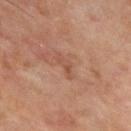This lesion was catalogued during total-body skin photography and was not selected for biopsy. This is a cross-polarized tile. A male subject roughly 55 years of age. The recorded lesion diameter is about 3.5 mm. The lesion is located on the chest. A roughly 15 mm field-of-view crop from a total-body skin photograph.